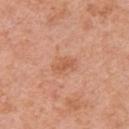subject = male, in their 70s; imaging modality = ~15 mm crop, total-body skin-cancer survey; illumination = white-light; anatomic site = the chest.